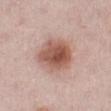{
  "biopsy_status": "not biopsied; imaged during a skin examination",
  "lighting": "white-light",
  "lesion_size": {
    "long_diameter_mm_approx": 5.5
  },
  "site": "left lower leg",
  "image": {
    "source": "total-body photography crop",
    "field_of_view_mm": 15
  },
  "automated_metrics": {
    "eccentricity": 0.6,
    "cielab_L": 55,
    "cielab_a": 22,
    "cielab_b": 26,
    "vs_skin_darker_L": 14.0,
    "vs_skin_contrast_norm": 9.5,
    "border_irregularity_0_10": 1.5,
    "color_variation_0_10": 6.5,
    "lesion_detection_confidence_0_100": 100
  },
  "patient": {
    "sex": "female",
    "age_approx": 30
  }
}